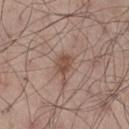This lesion was catalogued during total-body skin photography and was not selected for biopsy. Cropped from a total-body skin-imaging series; the visible field is about 15 mm. From the right thigh. A male subject roughly 55 years of age. This is a white-light tile. Automated image analysis of the tile measured an outline eccentricity of about 0.65 (0 = round, 1 = elongated) and two-axis asymmetry of about 0.25. The analysis additionally found a border-irregularity rating of about 2/10 and internal color variation of about 2.5 on a 0–10 scale. The analysis additionally found an automated nevus-likeness rating near 50 out of 100. About 2.5 mm across.